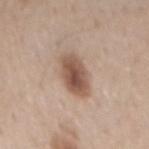* follow-up · imaged on a skin check; not biopsied
* lesion diameter · ≈5 mm
* tile lighting · white-light
* acquisition · ~15 mm crop, total-body skin-cancer survey
* patient · male, aged 58–62
* anatomic site · the back